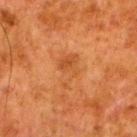Captured during whole-body skin photography for melanoma surveillance; the lesion was not biopsied.